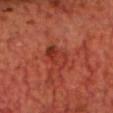Assessment:
Part of a total-body skin-imaging series; this lesion was reviewed on a skin check and was not flagged for biopsy.
Image and clinical context:
From the chest. The lesion-visualizer software estimated an average lesion color of about L≈36 a*≈33 b*≈32 (CIELAB), about 8 CIELAB-L* units darker than the surrounding skin, and a lesion-to-skin contrast of about 6.5 (normalized; higher = more distinct). And it measured a border-irregularity index near 2/10, a within-lesion color-variation index near 5.5/10, and peripheral color asymmetry of about 2. The tile uses cross-polarized illumination. A 15 mm crop from a total-body photograph taken for skin-cancer surveillance. The patient is a male about 70 years old. Approximately 3 mm at its widest.